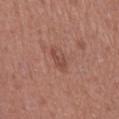Q: Was a biopsy performed?
A: no biopsy performed (imaged during a skin exam)
Q: What kind of image is this?
A: ~15 mm crop, total-body skin-cancer survey
Q: What is the anatomic site?
A: the right thigh
Q: Patient demographics?
A: female, in their 40s